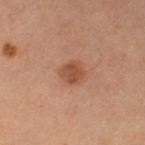<tbp_lesion>
  <biopsy_status>not biopsied; imaged during a skin examination</biopsy_status>
  <site>left thigh</site>
  <lighting>cross-polarized</lighting>
  <image>
    <source>total-body photography crop</source>
    <field_of_view_mm>15</field_of_view_mm>
  </image>
  <automated_metrics>
    <area_mm2_approx>5.5</area_mm2_approx>
    <shape_asymmetry>0.15</shape_asymmetry>
    <border_irregularity_0_10>1.5</border_irregularity_0_10>
    <color_variation_0_10>1.5</color_variation_0_10>
    <peripheral_color_asymmetry>0.5</peripheral_color_asymmetry>
  </automated_metrics>
  <patient>
    <sex>female</sex>
    <age_approx>55</age_approx>
  </patient>
</tbp_lesion>